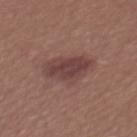The lesion was tiled from a total-body skin photograph and was not biopsied.
The lesion is on the mid back.
Longest diameter approximately 5.5 mm.
Cropped from a whole-body photographic skin survey; the tile spans about 15 mm.
The subject is a female in their mid- to late 20s.
The tile uses white-light illumination.